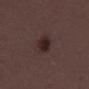size = ~3.5 mm (longest diameter); tile lighting = white-light; patient = male, about 50 years old; image = ~15 mm crop, total-body skin-cancer survey.